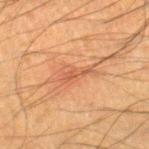<record>
<biopsy_status>not biopsied; imaged during a skin examination</biopsy_status>
<site>right lower leg</site>
<lighting>cross-polarized</lighting>
<lesion_size>
  <long_diameter_mm_approx>4.5</long_diameter_mm_approx>
</lesion_size>
<automated_metrics>
  <eccentricity>0.9</eccentricity>
  <shape_asymmetry>0.5</shape_asymmetry>
  <cielab_L>46</cielab_L>
  <cielab_a>21</cielab_a>
  <cielab_b>31</cielab_b>
  <vs_skin_darker_L>7.0</vs_skin_darker_L>
  <vs_skin_contrast_norm>5.5</vs_skin_contrast_norm>
  <color_variation_0_10>2.0</color_variation_0_10>
</automated_metrics>
<patient>
  <sex>male</sex>
  <age_approx>35</age_approx>
</patient>
<image>
  <source>total-body photography crop</source>
  <field_of_view_mm>15</field_of_view_mm>
</image>
</record>